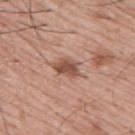Part of a total-body skin-imaging series; this lesion was reviewed on a skin check and was not flagged for biopsy.
A male subject aged around 55.
A roughly 15 mm field-of-view crop from a total-body skin photograph.